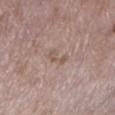{
  "biopsy_status": "not biopsied; imaged during a skin examination",
  "lighting": "white-light",
  "patient": {
    "sex": "female",
    "age_approx": 70
  },
  "automated_metrics": {
    "color_variation_0_10": 0.0,
    "peripheral_color_asymmetry": 0.0,
    "nevus_likeness_0_100": 0,
    "lesion_detection_confidence_0_100": 100
  },
  "site": "left lower leg",
  "image": {
    "source": "total-body photography crop",
    "field_of_view_mm": 15
  }
}